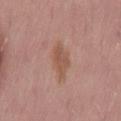follow-up=no biopsy performed (imaged during a skin exam); lesion size=about 4.5 mm; subject=male, aged around 55; lighting=white-light illumination; site=the lower back; acquisition=~15 mm crop, total-body skin-cancer survey; TBP lesion metrics=a classifier nevus-likeness of about 5/100.